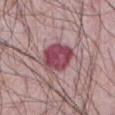* biopsy status · catalogued during a skin exam; not biopsied
* patient · male, in their mid- to late 60s
* illumination · white-light illumination
* image source · ~15 mm crop, total-body skin-cancer survey
* site · the abdomen
* lesion size · ~4.5 mm (longest diameter)